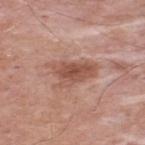Q: How was the tile lit?
A: white-light illumination
Q: Where on the body is the lesion?
A: the upper back
Q: Lesion size?
A: ~5.5 mm (longest diameter)
Q: What is the imaging modality?
A: 15 mm crop, total-body photography
Q: Patient demographics?
A: male, aged around 55